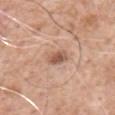The lesion was photographed on a routine skin check and not biopsied; there is no pathology result. On the right upper arm. A male patient aged 58 to 62. The lesion's longest dimension is about 3 mm. Cropped from a whole-body photographic skin survey; the tile spans about 15 mm. Automated image analysis of the tile measured a lesion area of about 4.5 mm², an eccentricity of roughly 0.8, and two-axis asymmetry of about 0.3. The software also gave a detector confidence of about 100 out of 100 that the crop contains a lesion. The tile uses white-light illumination.